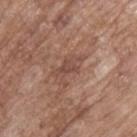The lesion was tiled from a total-body skin photograph and was not biopsied.
A 15 mm close-up tile from a total-body photography series done for melanoma screening.
The recorded lesion diameter is about 4 mm.
This is a white-light tile.
A male patient aged approximately 70.
Located on the back.